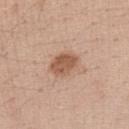follow-up: catalogued during a skin exam; not biopsied | subject: female, roughly 40 years of age | body site: the left upper arm | lesion size: ≈3.5 mm | image source: total-body-photography crop, ~15 mm field of view.